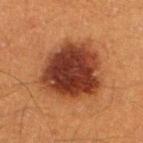Assessment:
Part of a total-body skin-imaging series; this lesion was reviewed on a skin check and was not flagged for biopsy.
Image and clinical context:
From the left lower leg. A male subject aged around 40. About 6.5 mm across. Imaged with cross-polarized lighting. A 15 mm crop from a total-body photograph taken for skin-cancer surveillance.